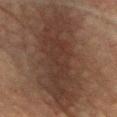Captured during whole-body skin photography for melanoma surveillance; the lesion was not biopsied. A 15 mm close-up tile from a total-body photography series done for melanoma screening. The patient is a male aged 58 to 62. On the front of the torso.